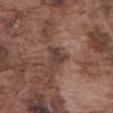notes: imaged on a skin check; not biopsied | lighting: white-light | image-analysis metrics: a footprint of about 4 mm², an outline eccentricity of about 0.6 (0 = round, 1 = elongated), and a symmetry-axis asymmetry near 0.45; a lesion-to-skin contrast of about 8.5 (normalized; higher = more distinct); an automated nevus-likeness rating near 0 out of 100 and lesion-presence confidence of about 100/100 | patient: male, aged around 75 | imaging modality: ~15 mm crop, total-body skin-cancer survey | body site: the abdomen.